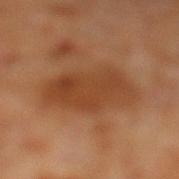Q: Is there a histopathology result?
A: imaged on a skin check; not biopsied
Q: Lesion location?
A: the left lower leg
Q: Lesion size?
A: ≈7 mm
Q: How was this image acquired?
A: total-body-photography crop, ~15 mm field of view
Q: Who is the patient?
A: male, approximately 60 years of age
Q: Illumination type?
A: cross-polarized illumination